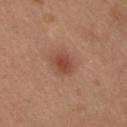Imaged during a routine full-body skin examination; the lesion was not biopsied and no histopathology is available.
A male subject aged 33 to 37.
The lesion is located on the upper back.
A 15 mm crop from a total-body photograph taken for skin-cancer surveillance.
Imaged with cross-polarized lighting.
The lesion's longest dimension is about 2.5 mm.
An algorithmic analysis of the crop reported a mean CIELAB color near L≈43 a*≈23 b*≈28 and a normalized lesion–skin contrast near 7.5.